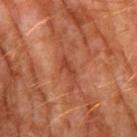Assessment: Imaged during a routine full-body skin examination; the lesion was not biopsied and no histopathology is available. Background: A male patient approximately 60 years of age. A region of skin cropped from a whole-body photographic capture, roughly 15 mm wide. Located on the right upper arm. Imaged with cross-polarized lighting.